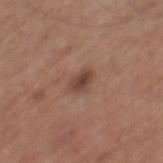follow-up: no biopsy performed (imaged during a skin exam)
acquisition: ~15 mm crop, total-body skin-cancer survey
lighting: white-light
diameter: ≈2.5 mm
anatomic site: the mid back
subject: male, in their mid-60s
TBP lesion metrics: a color-variation rating of about 3/10 and peripheral color asymmetry of about 1; an automated nevus-likeness rating near 80 out of 100 and lesion-presence confidence of about 100/100